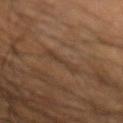acquisition = total-body-photography crop, ~15 mm field of view | anatomic site = the left forearm | tile lighting = cross-polarized illumination | subject = male, aged around 60 | diameter = ~3.5 mm (longest diameter).